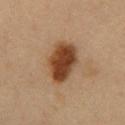Part of a total-body skin-imaging series; this lesion was reviewed on a skin check and was not flagged for biopsy. On the abdomen. Imaged with cross-polarized lighting. Longest diameter approximately 5.5 mm. This image is a 15 mm lesion crop taken from a total-body photograph. The patient is a male aged approximately 50.